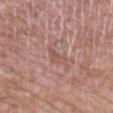Image and clinical context:
Imaged with white-light lighting. A roughly 15 mm field-of-view crop from a total-body skin photograph. A female subject about 70 years old. Approximately 3 mm at its widest. Automated tile analysis of the lesion measured a border-irregularity rating of about 5/10, a color-variation rating of about 1/10, and peripheral color asymmetry of about 0.5. The software also gave a nevus-likeness score of about 0/100 and lesion-presence confidence of about 90/100. The lesion is located on the left upper arm.